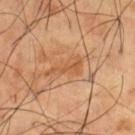biopsy status: total-body-photography surveillance lesion; no biopsy
diameter: ≈4.5 mm
image: ~15 mm tile from a whole-body skin photo
image-analysis metrics: a footprint of about 6 mm², an outline eccentricity of about 0.9 (0 = round, 1 = elongated), and a symmetry-axis asymmetry near 0.6
body site: the right thigh
patient: male, aged approximately 65